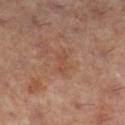Captured during whole-body skin photography for melanoma surveillance; the lesion was not biopsied. A 15 mm close-up tile from a total-body photography series done for melanoma screening. The lesion's longest dimension is about 3 mm. The lesion-visualizer software estimated an area of roughly 2.5 mm² and a symmetry-axis asymmetry near 0.4. The analysis additionally found a normalized lesion–skin contrast near 4.5. The software also gave a color-variation rating of about 0/10 and peripheral color asymmetry of about 0. The analysis additionally found a classifier nevus-likeness of about 0/100. From the right lower leg. The subject is a female aged 58 to 62. The tile uses cross-polarized illumination.